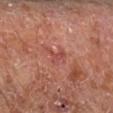Clinical impression: Part of a total-body skin-imaging series; this lesion was reviewed on a skin check and was not flagged for biopsy. Acquisition and patient details: The tile uses cross-polarized illumination. A 15 mm crop from a total-body photograph taken for skin-cancer surveillance. The subject is in their mid- to late 60s. The lesion is on the right lower leg. Measured at roughly 2.5 mm in maximum diameter.